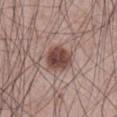About 3.5 mm across.
Located on the abdomen.
The tile uses white-light illumination.
A male subject aged 63 to 67.
Cropped from a total-body skin-imaging series; the visible field is about 15 mm.